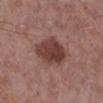workup: imaged on a skin check; not biopsied
body site: the right lower leg
lighting: white-light
subject: male, aged 68–72
TBP lesion metrics: a mean CIELAB color near L≈40 a*≈22 b*≈22 and roughly 12 lightness units darker than nearby skin; a within-lesion color-variation index near 4/10 and radial color variation of about 1.5
image source: total-body-photography crop, ~15 mm field of view